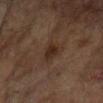Impression: Imaged during a routine full-body skin examination; the lesion was not biopsied and no histopathology is available. Context: A lesion tile, about 15 mm wide, cut from a 3D total-body photograph. The subject is a female about 80 years old. On the right arm.